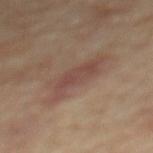workup: catalogued during a skin exam; not biopsied | subject: male, aged approximately 85 or older | lighting: cross-polarized illumination | site: the mid back | lesion diameter: ~6.5 mm (longest diameter) | image source: ~15 mm tile from a whole-body skin photo.